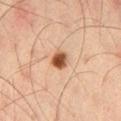No biopsy was performed on this lesion — it was imaged during a full skin examination and was not determined to be concerning.
The lesion is located on the left thigh.
The subject is a male aged around 35.
Automated tile analysis of the lesion measured an average lesion color of about L≈49 a*≈23 b*≈33 (CIELAB), about 17 CIELAB-L* units darker than the surrounding skin, and a normalized lesion–skin contrast near 12. And it measured a classifier nevus-likeness of about 100/100 and a detector confidence of about 100 out of 100 that the crop contains a lesion.
A lesion tile, about 15 mm wide, cut from a 3D total-body photograph.
This is a cross-polarized tile.